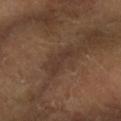Part of a total-body skin-imaging series; this lesion was reviewed on a skin check and was not flagged for biopsy.
This image is a 15 mm lesion crop taken from a total-body photograph.
This is a cross-polarized tile.
The lesion's longest dimension is about 4 mm.
The total-body-photography lesion software estimated an area of roughly 6 mm², a shape eccentricity near 0.95, and two-axis asymmetry of about 0.3. The software also gave internal color variation of about 1 on a 0–10 scale. And it measured a nevus-likeness score of about 0/100 and lesion-presence confidence of about 65/100.
Located on the right forearm.
The patient is approximately 60 years of age.